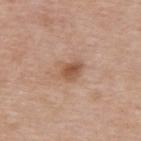The lesion was tiled from a total-body skin photograph and was not biopsied. The total-body-photography lesion software estimated a mean CIELAB color near L≈55 a*≈20 b*≈31, a lesion–skin lightness drop of about 10, and a normalized border contrast of about 7.5. The software also gave an automated nevus-likeness rating near 70 out of 100 and a detector confidence of about 100 out of 100 that the crop contains a lesion. The patient is a male in their mid-50s. On the upper back. Measured at roughly 3 mm in maximum diameter. A 15 mm close-up tile from a total-body photography series done for melanoma screening.